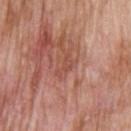Q: Was a biopsy performed?
A: imaged on a skin check; not biopsied
Q: Lesion size?
A: about 3.5 mm
Q: What lighting was used for the tile?
A: white-light
Q: Patient demographics?
A: male, in their 60s
Q: What kind of image is this?
A: 15 mm crop, total-body photography
Q: Lesion location?
A: the upper back
Q: What did automated image analysis measure?
A: a footprint of about 3.5 mm², a shape eccentricity near 0.9, and a symmetry-axis asymmetry near 0.6; a lesion color around L≈50 a*≈25 b*≈28 in CIELAB, roughly 7 lightness units darker than nearby skin, and a normalized lesion–skin contrast near 5; a border-irregularity rating of about 7/10, internal color variation of about 1 on a 0–10 scale, and peripheral color asymmetry of about 0.5; a nevus-likeness score of about 0/100 and lesion-presence confidence of about 65/100